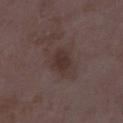| key | value |
|---|---|
| biopsy status | catalogued during a skin exam; not biopsied |
| image source | total-body-photography crop, ~15 mm field of view |
| lighting | white-light illumination |
| patient | female, approximately 35 years of age |
| diameter | about 3 mm |
| body site | the right thigh |